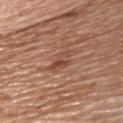Impression:
The lesion was tiled from a total-body skin photograph and was not biopsied.